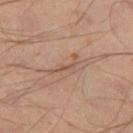Clinical impression:
Imaged during a routine full-body skin examination; the lesion was not biopsied and no histopathology is available.
Clinical summary:
A 15 mm close-up extracted from a 3D total-body photography capture. Located on the leg. The subject is a male aged 43–47. The lesion-visualizer software estimated a detector confidence of about 70 out of 100 that the crop contains a lesion. Measured at roughly 4 mm in maximum diameter.